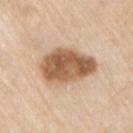{
  "biopsy_status": "not biopsied; imaged during a skin examination",
  "image": {
    "source": "total-body photography crop",
    "field_of_view_mm": 15
  },
  "patient": {
    "sex": "male",
    "age_approx": 80
  },
  "site": "left upper arm",
  "automated_metrics": {
    "nevus_likeness_0_100": 60
  }
}